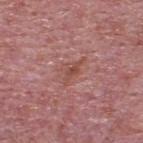No biopsy was performed on this lesion — it was imaged during a full skin examination and was not determined to be concerning. The lesion is on the upper back. A roughly 15 mm field-of-view crop from a total-body skin photograph. The subject is a male in their mid-70s.